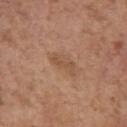biopsy status: catalogued during a skin exam; not biopsied | acquisition: total-body-photography crop, ~15 mm field of view | subject: female, aged approximately 65 | body site: the left upper arm.